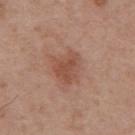Assessment: Part of a total-body skin-imaging series; this lesion was reviewed on a skin check and was not flagged for biopsy. Image and clinical context: Captured under white-light illumination. The total-body-photography lesion software estimated a lesion area of about 9 mm², a shape eccentricity near 0.55, and a shape-asymmetry score of about 0.3 (0 = symmetric). The software also gave border irregularity of about 3 on a 0–10 scale, internal color variation of about 3 on a 0–10 scale, and a peripheral color-asymmetry measure near 1. It also reported a nevus-likeness score of about 55/100 and a lesion-detection confidence of about 100/100. On the upper back. About 4 mm across. The subject is a male aged approximately 55. A lesion tile, about 15 mm wide, cut from a 3D total-body photograph.